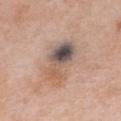Captured during whole-body skin photography for melanoma surveillance; the lesion was not biopsied. A 15 mm close-up tile from a total-body photography series done for melanoma screening. On the chest. This is a white-light tile. Longest diameter approximately 6 mm. A female subject, aged 33 to 37.